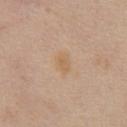<record>
  <biopsy_status>not biopsied; imaged during a skin examination</biopsy_status>
  <lesion_size>
    <long_diameter_mm_approx>2.5</long_diameter_mm_approx>
  </lesion_size>
  <image>
    <source>total-body photography crop</source>
    <field_of_view_mm>15</field_of_view_mm>
  </image>
  <patient>
    <sex>female</sex>
    <age_approx>70</age_approx>
  </patient>
  <site>chest</site>
  <lighting>white-light</lighting>
</record>